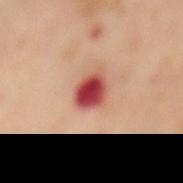Clinical impression:
Recorded during total-body skin imaging; not selected for excision or biopsy.
Image and clinical context:
Located on the back. This image is a 15 mm lesion crop taken from a total-body photograph. The patient is a female aged 53 to 57.